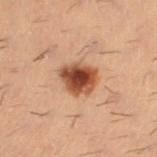workup: imaged on a skin check; not biopsied | illumination: cross-polarized illumination | anatomic site: the right thigh | patient: male, approximately 30 years of age | image source: total-body-photography crop, ~15 mm field of view | diameter: ~4 mm (longest diameter).